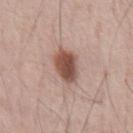notes: no biopsy performed (imaged during a skin exam) | patient: male, aged approximately 55 | illumination: white-light illumination | diameter: about 4 mm | imaging modality: ~15 mm crop, total-body skin-cancer survey.